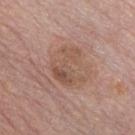Findings:
* follow-up: imaged on a skin check; not biopsied
* TBP lesion metrics: a border-irregularity rating of about 8.5/10
* subject: male, in their 60s
* anatomic site: the chest
* acquisition: ~15 mm tile from a whole-body skin photo
* illumination: white-light
* lesion size: about 4.5 mm